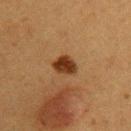Case summary:
* workup: imaged on a skin check; not biopsied
* subject: female, roughly 40 years of age
* body site: the left upper arm
* acquisition: 15 mm crop, total-body photography